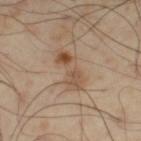Imaged during a routine full-body skin examination; the lesion was not biopsied and no histopathology is available. About 5 mm across. Imaged with cross-polarized lighting. The subject is a male roughly 55 years of age. The lesion is located on the left thigh. A 15 mm close-up extracted from a 3D total-body photography capture. Automated tile analysis of the lesion measured a footprint of about 9 mm². The software also gave an automated nevus-likeness rating near 45 out of 100.